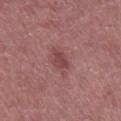workup: imaged on a skin check; not biopsied | automated metrics: an average lesion color of about L≈44 a*≈25 b*≈21 (CIELAB) and a normalized lesion–skin contrast near 6.5 | site: the mid back | tile lighting: white-light | image source: total-body-photography crop, ~15 mm field of view | size: ≈2.5 mm | subject: male, aged 58 to 62.